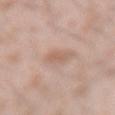Recorded during total-body skin imaging; not selected for excision or biopsy. A male subject roughly 25 years of age. The lesion-visualizer software estimated a shape eccentricity near 0.95. The analysis additionally found an average lesion color of about L≈59 a*≈19 b*≈28 (CIELAB), a lesion–skin lightness drop of about 8, and a lesion-to-skin contrast of about 5.5 (normalized; higher = more distinct). The analysis additionally found an automated nevus-likeness rating near 0 out of 100 and a detector confidence of about 100 out of 100 that the crop contains a lesion. About 3 mm across. A lesion tile, about 15 mm wide, cut from a 3D total-body photograph. This is a white-light tile. From the right forearm.